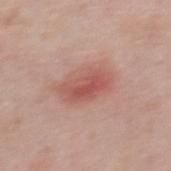follow-up — no biopsy performed (imaged during a skin exam)
patient — male, roughly 40 years of age
body site — the mid back
illumination — white-light illumination
automated metrics — an outline eccentricity of about 0.8 (0 = round, 1 = elongated) and two-axis asymmetry of about 0.15; a color-variation rating of about 5/10 and a peripheral color-asymmetry measure near 1.5; a classifier nevus-likeness of about 25/100 and a lesion-detection confidence of about 100/100
imaging modality — ~15 mm tile from a whole-body skin photo